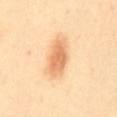follow-up = total-body-photography surveillance lesion; no biopsy.